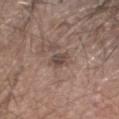Imaged during a routine full-body skin examination; the lesion was not biopsied and no histopathology is available.
Located on the head or neck.
Imaged with white-light lighting.
The patient is a male aged 23–27.
A 15 mm close-up tile from a total-body photography series done for melanoma screening.
Longest diameter approximately 3.5 mm.